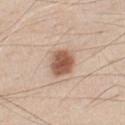* follow-up — catalogued during a skin exam; not biopsied
* anatomic site — the chest
* TBP lesion metrics — an average lesion color of about L≈56 a*≈20 b*≈30 (CIELAB), about 15 CIELAB-L* units darker than the surrounding skin, and a lesion-to-skin contrast of about 10 (normalized; higher = more distinct); a border-irregularity index near 1.5/10, internal color variation of about 4 on a 0–10 scale, and peripheral color asymmetry of about 1; a lesion-detection confidence of about 100/100
* subject — male, approximately 45 years of age
* imaging modality — total-body-photography crop, ~15 mm field of view
* diameter — ≈3.5 mm
* illumination — white-light illumination